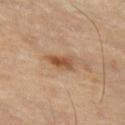{
  "image": {
    "source": "total-body photography crop",
    "field_of_view_mm": 15
  },
  "site": "left thigh",
  "patient": {
    "sex": "female",
    "age_approx": 50
  }
}